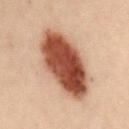{"biopsy_status": "not biopsied; imaged during a skin examination"}